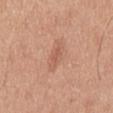Q: Is there a histopathology result?
A: no biopsy performed (imaged during a skin exam)
Q: What is the lesion's diameter?
A: about 4 mm
Q: How was the tile lit?
A: white-light illumination
Q: How was this image acquired?
A: 15 mm crop, total-body photography
Q: Where on the body is the lesion?
A: the mid back
Q: What are the patient's age and sex?
A: male, aged around 30